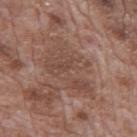Part of a total-body skin-imaging series; this lesion was reviewed on a skin check and was not flagged for biopsy.
About 7.5 mm across.
Imaged with white-light lighting.
A male patient, roughly 70 years of age.
A 15 mm close-up tile from a total-body photography series done for melanoma screening.
The lesion is located on the mid back.